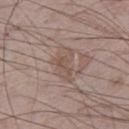No biopsy was performed on this lesion — it was imaged during a full skin examination and was not determined to be concerning.
A male patient, aged 73 to 77.
The lesion is on the leg.
Cropped from a total-body skin-imaging series; the visible field is about 15 mm.
Automated tile analysis of the lesion measured an average lesion color of about L≈52 a*≈15 b*≈23 (CIELAB), roughly 7 lightness units darker than nearby skin, and a normalized border contrast of about 5.5.